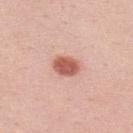Impression:
No biopsy was performed on this lesion — it was imaged during a full skin examination and was not determined to be concerning.
Context:
About 3 mm across. A 15 mm crop from a total-body photograph taken for skin-cancer surveillance. Imaged with white-light lighting. The total-body-photography lesion software estimated a symmetry-axis asymmetry near 0.2. It also reported internal color variation of about 2.5 on a 0–10 scale and a peripheral color-asymmetry measure near 1. A male subject in their mid- to late 30s. From the upper back.